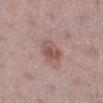Findings:
• follow-up · imaged on a skin check; not biopsied
• location · the right lower leg
• size · ≈3.5 mm
• imaging modality · total-body-photography crop, ~15 mm field of view
• automated metrics · an area of roughly 7 mm² and a shape eccentricity near 0.7; a lesion color around L≈53 a*≈20 b*≈23 in CIELAB, about 9 CIELAB-L* units darker than the surrounding skin, and a lesion-to-skin contrast of about 7 (normalized; higher = more distinct)
• patient · female, in their mid-40s